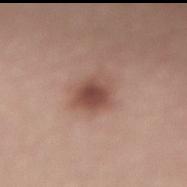workup: imaged on a skin check; not biopsied
diameter: about 3.5 mm
subject: male, aged 48 to 52
tile lighting: white-light illumination
anatomic site: the back
acquisition: ~15 mm crop, total-body skin-cancer survey